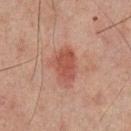  biopsy_status: not biopsied; imaged during a skin examination
  automated_metrics:
    cielab_L: 40
    cielab_a: 22
    cielab_b: 24
    vs_skin_darker_L: 8.0
    vs_skin_contrast_norm: 7.0
  site: mid back
  image:
    source: total-body photography crop
    field_of_view_mm: 15
  patient:
    sex: male
    age_approx: 65
  lighting: cross-polarized
  lesion_size:
    long_diameter_mm_approx: 4.5